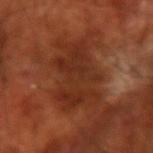| feature | finding |
|---|---|
| follow-up | catalogued during a skin exam; not biopsied |
| TBP lesion metrics | a lesion area of about 22 mm², a shape eccentricity near 0.85, and a shape-asymmetry score of about 0.35 (0 = symmetric); a mean CIELAB color near L≈29 a*≈25 b*≈30 |
| image | ~15 mm tile from a whole-body skin photo |
| patient | male, approximately 70 years of age |
| body site | the right forearm |